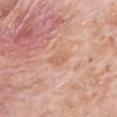| field | value |
|---|---|
| follow-up | imaged on a skin check; not biopsied |
| patient | male, aged 78–82 |
| size | about 3 mm |
| lighting | white-light illumination |
| location | the left upper arm |
| imaging modality | total-body-photography crop, ~15 mm field of view |